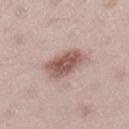Impression:
No biopsy was performed on this lesion — it was imaged during a full skin examination and was not determined to be concerning.
Context:
The subject is a female approximately 25 years of age. From the right lower leg. This image is a 15 mm lesion crop taken from a total-body photograph.